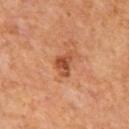illumination — cross-polarized illumination
subject — male, in their mid- to late 60s
size — ~3 mm (longest diameter)
automated lesion analysis — a footprint of about 4.5 mm², a shape eccentricity near 0.7, and a shape-asymmetry score of about 0.4 (0 = symmetric); roughly 11 lightness units darker than nearby skin
site — the back
imaging modality — 15 mm crop, total-body photography